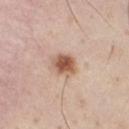Clinical impression: The lesion was photographed on a routine skin check and not biopsied; there is no pathology result. Clinical summary: The lesion is located on the chest. Cropped from a whole-body photographic skin survey; the tile spans about 15 mm. A male patient, in their mid-40s. The recorded lesion diameter is about 3 mm. The tile uses white-light illumination.